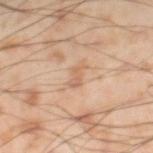The lesion was tiled from a total-body skin photograph and was not biopsied.
An algorithmic analysis of the crop reported an area of roughly 3 mm², an eccentricity of roughly 0.85, and a shape-asymmetry score of about 0.45 (0 = symmetric). It also reported an average lesion color of about L≈63 a*≈19 b*≈33 (CIELAB), about 8 CIELAB-L* units darker than the surrounding skin, and a lesion-to-skin contrast of about 5 (normalized; higher = more distinct). It also reported a border-irregularity index near 4.5/10, a color-variation rating of about 0/10, and peripheral color asymmetry of about 0.
The lesion is on the left thigh.
Approximately 2.5 mm at its widest.
A 15 mm crop from a total-body photograph taken for skin-cancer surveillance.
The patient is a male approximately 55 years of age.